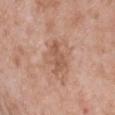Q: Was this lesion biopsied?
A: no biopsy performed (imaged during a skin exam)
Q: What is the anatomic site?
A: the chest
Q: What are the patient's age and sex?
A: male, in their 50s
Q: How was the tile lit?
A: white-light
Q: How was this image acquired?
A: ~15 mm tile from a whole-body skin photo
Q: What did automated image analysis measure?
A: border irregularity of about 4.5 on a 0–10 scale and a color-variation rating of about 2/10; an automated nevus-likeness rating near 0 out of 100 and lesion-presence confidence of about 100/100
Q: What is the lesion's diameter?
A: about 3.5 mm